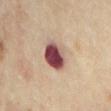Notes:
* workup: imaged on a skin check; not biopsied